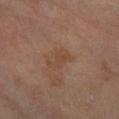Part of a total-body skin-imaging series; this lesion was reviewed on a skin check and was not flagged for biopsy. This is a cross-polarized tile. A 15 mm close-up extracted from a 3D total-body photography capture. A female patient, approximately 65 years of age. Located on the left leg.